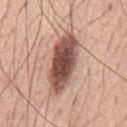notes — total-body-photography surveillance lesion; no biopsy | patient — male, aged 58–62 | image source — ~15 mm crop, total-body skin-cancer survey | body site — the mid back | diameter — ~8 mm (longest diameter).